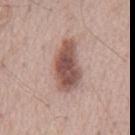biopsy_status: not biopsied; imaged during a skin examination
site: back
lighting: white-light
image:
  source: total-body photography crop
  field_of_view_mm: 15
automated_metrics:
  area_mm2_approx: 16.0
  eccentricity: 0.9
  shape_asymmetry: 0.25
  border_irregularity_0_10: 3.0
  color_variation_0_10: 4.5
  peripheral_color_asymmetry: 1.5
  lesion_detection_confidence_0_100: 100
patient:
  sex: male
  age_approx: 65
lesion_size:
  long_diameter_mm_approx: 7.0